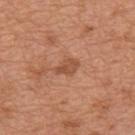Q: Was this lesion biopsied?
A: catalogued during a skin exam; not biopsied
Q: What is the lesion's diameter?
A: ≈3 mm
Q: What did automated image analysis measure?
A: an area of roughly 3.5 mm², an outline eccentricity of about 0.8 (0 = round, 1 = elongated), and a symmetry-axis asymmetry near 0.25; a color-variation rating of about 2/10 and peripheral color asymmetry of about 0.5
Q: What lighting was used for the tile?
A: white-light illumination
Q: What is the anatomic site?
A: the mid back
Q: How was this image acquired?
A: total-body-photography crop, ~15 mm field of view
Q: Who is the patient?
A: male, aged 63 to 67